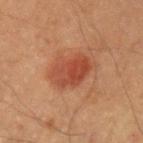Notes:
• notes: catalogued during a skin exam; not biopsied
• lighting: cross-polarized illumination
• size: ~4.5 mm (longest diameter)
• image-analysis metrics: an average lesion color of about L≈42 a*≈26 b*≈31 (CIELAB), about 9 CIELAB-L* units darker than the surrounding skin, and a lesion-to-skin contrast of about 7.5 (normalized; higher = more distinct); internal color variation of about 4.5 on a 0–10 scale and peripheral color asymmetry of about 1.5
• image: 15 mm crop, total-body photography
• subject: male, about 40 years old
• anatomic site: the arm